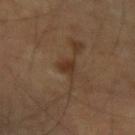The lesion was photographed on a routine skin check and not biopsied; there is no pathology result. The subject is a male roughly 65 years of age. The lesion is located on the left forearm. Captured under cross-polarized illumination. Longest diameter approximately 2.5 mm. A roughly 15 mm field-of-view crop from a total-body skin photograph.